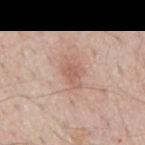Notes:
* notes — imaged on a skin check; not biopsied
* imaging modality — 15 mm crop, total-body photography
* location — the mid back
* patient — male, approximately 55 years of age
* tile lighting — white-light